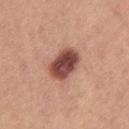notes: total-body-photography surveillance lesion; no biopsy | lighting: white-light | anatomic site: the left thigh | patient: female, aged approximately 25 | diameter: ~4.5 mm (longest diameter) | acquisition: total-body-photography crop, ~15 mm field of view.